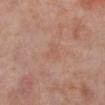Q: Was a biopsy performed?
A: catalogued during a skin exam; not biopsied
Q: What kind of image is this?
A: ~15 mm tile from a whole-body skin photo
Q: Patient demographics?
A: female, aged 48–52
Q: Where on the body is the lesion?
A: the left lower leg